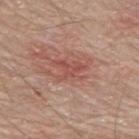Q: Was a biopsy performed?
A: imaged on a skin check; not biopsied
Q: What lighting was used for the tile?
A: white-light
Q: What are the patient's age and sex?
A: male, aged 68–72
Q: Automated lesion metrics?
A: a symmetry-axis asymmetry near 0.45; a lesion color around L≈51 a*≈26 b*≈26 in CIELAB, about 8 CIELAB-L* units darker than the surrounding skin, and a lesion-to-skin contrast of about 6 (normalized; higher = more distinct); a border-irregularity rating of about 7/10 and a peripheral color-asymmetry measure near 0.5
Q: What is the imaging modality?
A: 15 mm crop, total-body photography
Q: How large is the lesion?
A: ≈4 mm
Q: Lesion location?
A: the back